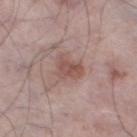Assessment:
This lesion was catalogued during total-body skin photography and was not selected for biopsy.
Clinical summary:
Automated tile analysis of the lesion measured an area of roughly 6 mm², a shape eccentricity near 0.75, and two-axis asymmetry of about 0.4. The software also gave a classifier nevus-likeness of about 40/100 and a lesion-detection confidence of about 100/100. A male subject, approximately 65 years of age. Approximately 3.5 mm at its widest. Cropped from a whole-body photographic skin survey; the tile spans about 15 mm. The lesion is on the left thigh.